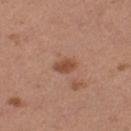Q: Was this lesion biopsied?
A: no biopsy performed (imaged during a skin exam)
Q: What is the imaging modality?
A: ~15 mm crop, total-body skin-cancer survey
Q: Lesion location?
A: the left thigh
Q: Patient demographics?
A: female, aged around 30
Q: How large is the lesion?
A: ≈2.5 mm